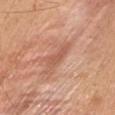<tbp_lesion>
<biopsy_status>not biopsied; imaged during a skin examination</biopsy_status>
</tbp_lesion>